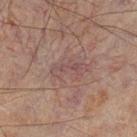No biopsy was performed on this lesion — it was imaged during a full skin examination and was not determined to be concerning.
The lesion is located on the left lower leg.
Measured at roughly 4 mm in maximum diameter.
An algorithmic analysis of the crop reported a lesion area of about 5 mm², a shape eccentricity near 0.95, and two-axis asymmetry of about 0.5. The software also gave a mean CIELAB color near L≈35 a*≈14 b*≈16 and roughly 5 lightness units darker than nearby skin. And it measured border irregularity of about 6.5 on a 0–10 scale, internal color variation of about 0 on a 0–10 scale, and a peripheral color-asymmetry measure near 0. It also reported an automated nevus-likeness rating near 0 out of 100 and a detector confidence of about 80 out of 100 that the crop contains a lesion.
The patient is a male aged approximately 60.
Cropped from a total-body skin-imaging series; the visible field is about 15 mm.